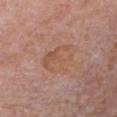Captured during whole-body skin photography for melanoma surveillance; the lesion was not biopsied. The lesion is on the chest. Cropped from a whole-body photographic skin survey; the tile spans about 15 mm. A male patient in their 80s.